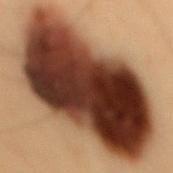This lesion was catalogued during total-body skin photography and was not selected for biopsy.